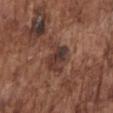Clinical impression:
Recorded during total-body skin imaging; not selected for excision or biopsy.
Background:
A male subject approximately 75 years of age. The total-body-photography lesion software estimated a footprint of about 11 mm², a shape eccentricity near 0.55, and two-axis asymmetry of about 0.3. And it measured border irregularity of about 4 on a 0–10 scale, a color-variation rating of about 7/10, and a peripheral color-asymmetry measure near 2.5. Imaged with white-light lighting. A lesion tile, about 15 mm wide, cut from a 3D total-body photograph. On the chest. The recorded lesion diameter is about 4 mm.